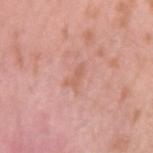Case summary:
– lighting: white-light illumination
– patient: male, aged approximately 25
– image-analysis metrics: a footprint of about 2 mm², a shape eccentricity near 0.9, and a shape-asymmetry score of about 0.4 (0 = symmetric); a mean CIELAB color near L≈61 a*≈25 b*≈30 and a lesion–skin lightness drop of about 7; border irregularity of about 5 on a 0–10 scale, internal color variation of about 0 on a 0–10 scale, and peripheral color asymmetry of about 0
– imaging modality: ~15 mm crop, total-body skin-cancer survey
– lesion size: ≈2.5 mm
– location: the left upper arm